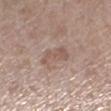{"biopsy_status": "not biopsied; imaged during a skin examination", "lighting": "white-light", "lesion_size": {"long_diameter_mm_approx": 4.0}, "site": "left lower leg", "patient": {"sex": "female", "age_approx": 55}, "image": {"source": "total-body photography crop", "field_of_view_mm": 15}}